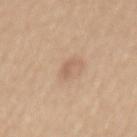Clinical impression: This lesion was catalogued during total-body skin photography and was not selected for biopsy. Background: Cropped from a whole-body photographic skin survey; the tile spans about 15 mm. On the mid back. A female patient, aged 53–57.